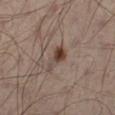The lesion was tiled from a total-body skin photograph and was not biopsied.
A 15 mm close-up tile from a total-body photography series done for melanoma screening.
From the left leg.
The lesion's longest dimension is about 3.5 mm.
The total-body-photography lesion software estimated a lesion area of about 5 mm². The analysis additionally found a classifier nevus-likeness of about 95/100 and a detector confidence of about 100 out of 100 that the crop contains a lesion.
The tile uses cross-polarized illumination.
The patient is a male aged approximately 50.